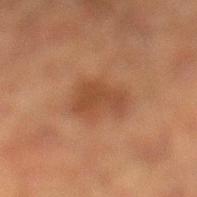  biopsy_status: not biopsied; imaged during a skin examination
  image:
    source: total-body photography crop
    field_of_view_mm: 15
  site: left lower leg
  lesion_size:
    long_diameter_mm_approx: 4.0
  patient:
    sex: male
    age_approx: 50
  lighting: cross-polarized